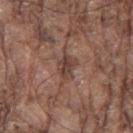Findings:
– follow-up: imaged on a skin check; not biopsied
– illumination: white-light
– body site: the left thigh
– patient: male, aged approximately 80
– imaging modality: 15 mm crop, total-body photography